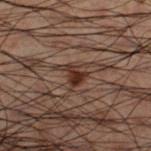| key | value |
|---|---|
| follow-up | imaged on a skin check; not biopsied |
| subject | male, aged 48–52 |
| size | ~2.5 mm (longest diameter) |
| location | the right lower leg |
| acquisition | ~15 mm crop, total-body skin-cancer survey |
| automated metrics | a lesion area of about 3.5 mm² and an outline eccentricity of about 0.7 (0 = round, 1 = elongated); a lesion color around L≈22 a*≈15 b*≈20 in CIELAB and a lesion-to-skin contrast of about 10.5 (normalized; higher = more distinct) |
| tile lighting | cross-polarized illumination |